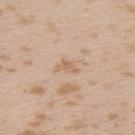Q: Was a biopsy performed?
A: no biopsy performed (imaged during a skin exam)
Q: What kind of image is this?
A: ~15 mm crop, total-body skin-cancer survey
Q: Where on the body is the lesion?
A: the left upper arm
Q: Who is the patient?
A: female, aged around 25